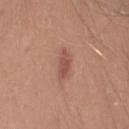Q: Was a biopsy performed?
A: no biopsy performed (imaged during a skin exam)
Q: Illumination type?
A: white-light illumination
Q: Automated lesion metrics?
A: a footprint of about 5 mm² and a shape-asymmetry score of about 0.25 (0 = symmetric)
Q: Patient demographics?
A: male, about 25 years old
Q: What is the anatomic site?
A: the arm
Q: What kind of image is this?
A: total-body-photography crop, ~15 mm field of view